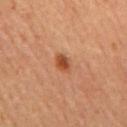Impression: Recorded during total-body skin imaging; not selected for excision or biopsy. Image and clinical context: Automated tile analysis of the lesion measured a shape eccentricity near 0.7 and a symmetry-axis asymmetry near 0.25. The software also gave an automated nevus-likeness rating near 95 out of 100 and a detector confidence of about 100 out of 100 that the crop contains a lesion. A male subject roughly 65 years of age. Imaged with cross-polarized lighting. A roughly 15 mm field-of-view crop from a total-body skin photograph. The lesion's longest dimension is about 2.5 mm. The lesion is on the back.